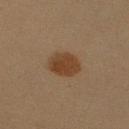Assessment: No biopsy was performed on this lesion — it was imaged during a full skin examination and was not determined to be concerning.